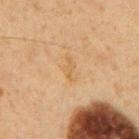{"biopsy_status": "not biopsied; imaged during a skin examination", "image": {"source": "total-body photography crop", "field_of_view_mm": 15}, "patient": {"sex": "male", "age_approx": 60}, "site": "mid back", "automated_metrics": {"area_mm2_approx": 2.0, "eccentricity": 0.95, "shape_asymmetry": 0.5}, "lighting": "cross-polarized", "lesion_size": {"long_diameter_mm_approx": 2.5}}